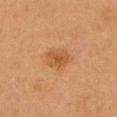Q: Was this lesion biopsied?
A: catalogued during a skin exam; not biopsied
Q: Patient demographics?
A: female, in their mid-40s
Q: Lesion location?
A: the head or neck
Q: What did automated image analysis measure?
A: a mean CIELAB color near L≈47 a*≈21 b*≈36 and about 7 CIELAB-L* units darker than the surrounding skin; border irregularity of about 2 on a 0–10 scale, internal color variation of about 3 on a 0–10 scale, and radial color variation of about 1; a classifier nevus-likeness of about 60/100 and lesion-presence confidence of about 100/100
Q: How large is the lesion?
A: about 3.5 mm
Q: What kind of image is this?
A: 15 mm crop, total-body photography
Q: Illumination type?
A: cross-polarized illumination